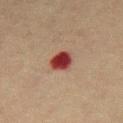{
  "biopsy_status": "not biopsied; imaged during a skin examination",
  "patient": {
    "sex": "male",
    "age_approx": 70
  },
  "site": "abdomen",
  "image": {
    "source": "total-body photography crop",
    "field_of_view_mm": 15
  },
  "lighting": "cross-polarized",
  "lesion_size": {
    "long_diameter_mm_approx": 3.0
  }
}